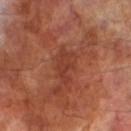Assessment: Part of a total-body skin-imaging series; this lesion was reviewed on a skin check and was not flagged for biopsy. Clinical summary: This is a cross-polarized tile. Automated image analysis of the tile measured a lesion area of about 7.5 mm² and an outline eccentricity of about 0.85 (0 = round, 1 = elongated). And it measured an average lesion color of about L≈38 a*≈28 b*≈30 (CIELAB), a lesion–skin lightness drop of about 7, and a lesion-to-skin contrast of about 6 (normalized; higher = more distinct). And it measured a border-irregularity rating of about 4.5/10, a within-lesion color-variation index near 2/10, and a peripheral color-asymmetry measure near 0.5. The analysis additionally found a nevus-likeness score of about 0/100 and lesion-presence confidence of about 100/100. A close-up tile cropped from a whole-body skin photograph, about 15 mm across. A male patient about 70 years old. The lesion is on the left lower leg. The lesion's longest dimension is about 4.5 mm.